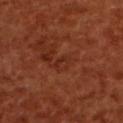This lesion was catalogued during total-body skin photography and was not selected for biopsy. Automated tile analysis of the lesion measured an eccentricity of roughly 0.95 and a shape-asymmetry score of about 0.5 (0 = symmetric). The analysis additionally found a border-irregularity rating of about 5/10 and a within-lesion color-variation index near 0/10. The analysis additionally found a nevus-likeness score of about 0/100. A roughly 15 mm field-of-view crop from a total-body skin photograph. A female patient in their mid-50s. Captured under cross-polarized illumination. On the upper back.